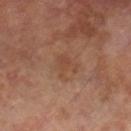Recorded during total-body skin imaging; not selected for excision or biopsy. Captured under cross-polarized illumination. The recorded lesion diameter is about 3 mm. On the right lower leg. A 15 mm crop from a total-body photograph taken for skin-cancer surveillance. A male subject, roughly 70 years of age.